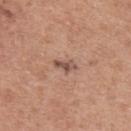Assessment:
Captured during whole-body skin photography for melanoma surveillance; the lesion was not biopsied.
Background:
Approximately 2.5 mm at its widest. The lesion is on the upper back. The subject is a female in their 60s. This image is a 15 mm lesion crop taken from a total-body photograph. Imaged with white-light lighting.